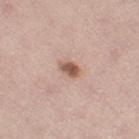Assessment: Part of a total-body skin-imaging series; this lesion was reviewed on a skin check and was not flagged for biopsy. Background: The recorded lesion diameter is about 2.5 mm. The total-body-photography lesion software estimated a lesion area of about 4 mm², an outline eccentricity of about 0.75 (0 = round, 1 = elongated), and two-axis asymmetry of about 0.2. It also reported a mean CIELAB color near L≈57 a*≈20 b*≈27, roughly 13 lightness units darker than nearby skin, and a lesion-to-skin contrast of about 8.5 (normalized; higher = more distinct). It also reported an automated nevus-likeness rating near 90 out of 100 and lesion-presence confidence of about 100/100. Captured under white-light illumination. The patient is a male aged 58–62. Located on the left thigh. This image is a 15 mm lesion crop taken from a total-body photograph.